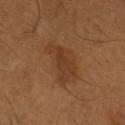Clinical summary: A male patient aged 63–67. Imaged with cross-polarized lighting. Longest diameter approximately 5.5 mm. The lesion is on the left forearm. A close-up tile cropped from a whole-body skin photograph, about 15 mm across. The lesion-visualizer software estimated an area of roughly 13 mm², an eccentricity of roughly 0.85, and a shape-asymmetry score of about 0.35 (0 = symmetric). The software also gave a border-irregularity rating of about 3.5/10.